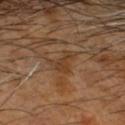Findings:
- biopsy status · no biopsy performed (imaged during a skin exam)
- illumination · cross-polarized illumination
- patient · male, in their 50s
- image source · ~15 mm tile from a whole-body skin photo
- size · ~3.5 mm (longest diameter)
- anatomic site · the head or neck